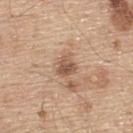Findings:
- notes · catalogued during a skin exam; not biopsied
- body site · the upper back
- acquisition · 15 mm crop, total-body photography
- subject · male, aged 68 to 72
- lesion diameter · ~3 mm (longest diameter)
- automated lesion analysis · an area of roughly 4.5 mm² and a shape eccentricity near 0.5; about 11 CIELAB-L* units darker than the surrounding skin and a normalized lesion–skin contrast near 7.5; radial color variation of about 1; lesion-presence confidence of about 100/100
- illumination · white-light illumination